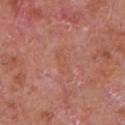<record>
<biopsy_status>not biopsied; imaged during a skin examination</biopsy_status>
<lighting>white-light</lighting>
<patient>
  <sex>male</sex>
  <age_approx>65</age_approx>
</patient>
<lesion_size>
  <long_diameter_mm_approx>3.0</long_diameter_mm_approx>
</lesion_size>
<site>chest</site>
<automated_metrics>
  <nevus_likeness_0_100>0</nevus_likeness_0_100>
  <lesion_detection_confidence_0_100>100</lesion_detection_confidence_0_100>
</automated_metrics>
<image>
  <source>total-body photography crop</source>
  <field_of_view_mm>15</field_of_view_mm>
</image>
</record>